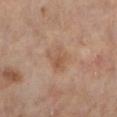The lesion's longest dimension is about 3.5 mm. A close-up tile cropped from a whole-body skin photograph, about 15 mm across. Imaged with cross-polarized lighting. Automated tile analysis of the lesion measured a border-irregularity index near 3.5/10 and a peripheral color-asymmetry measure near 1. The lesion is on the leg. The subject is a female aged 58 to 62.